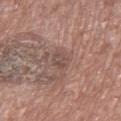workup: imaged on a skin check; not biopsied
image source: 15 mm crop, total-body photography
subject: female, aged around 75
tile lighting: white-light illumination
automated metrics: a lesion area of about 4 mm² and a symmetry-axis asymmetry near 0.2; a lesion color around L≈49 a*≈17 b*≈22 in CIELAB; a border-irregularity rating of about 2/10, internal color variation of about 3 on a 0–10 scale, and radial color variation of about 1
location: the left thigh
diameter: about 2.5 mm